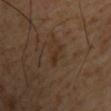A male patient aged approximately 50. The lesion is located on the back. Cropped from a whole-body photographic skin survey; the tile spans about 15 mm.